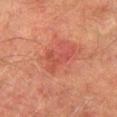Q: Was this lesion biopsied?
A: catalogued during a skin exam; not biopsied
Q: Illumination type?
A: cross-polarized illumination
Q: How large is the lesion?
A: about 4.5 mm
Q: What is the imaging modality?
A: 15 mm crop, total-body photography
Q: Patient demographics?
A: male, about 75 years old
Q: Where on the body is the lesion?
A: the left thigh